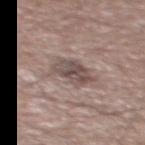The lesion was photographed on a routine skin check and not biopsied; there is no pathology result.
Located on the back.
The lesion-visualizer software estimated an outline eccentricity of about 0.7 (0 = round, 1 = elongated) and two-axis asymmetry of about 0.3. It also reported a mean CIELAB color near L≈49 a*≈14 b*≈18, about 11 CIELAB-L* units darker than the surrounding skin, and a normalized lesion–skin contrast near 8. The analysis additionally found a within-lesion color-variation index near 5/10 and a peripheral color-asymmetry measure near 1.5. And it measured an automated nevus-likeness rating near 0 out of 100 and a detector confidence of about 100 out of 100 that the crop contains a lesion.
This is a white-light tile.
Cropped from a whole-body photographic skin survey; the tile spans about 15 mm.
A male patient roughly 55 years of age.